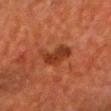<case>
  <biopsy_status>not biopsied; imaged during a skin examination</biopsy_status>
  <lighting>cross-polarized</lighting>
  <patient>
    <sex>male</sex>
    <age_approx>65</age_approx>
  </patient>
  <site>chest</site>
  <image>
    <source>total-body photography crop</source>
    <field_of_view_mm>15</field_of_view_mm>
  </image>
  <lesion_size>
    <long_diameter_mm_approx>4.5</long_diameter_mm_approx>
  </lesion_size>
  <automated_metrics>
    <nevus_likeness_0_100>15</nevus_likeness_0_100>
  </automated_metrics>
</case>